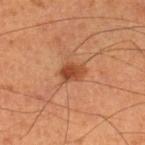Imaged during a routine full-body skin examination; the lesion was not biopsied and no histopathology is available. Approximately 3 mm at its widest. The tile uses cross-polarized illumination. A lesion tile, about 15 mm wide, cut from a 3D total-body photograph. Automated tile analysis of the lesion measured an average lesion color of about L≈48 a*≈27 b*≈37 (CIELAB) and a lesion-to-skin contrast of about 8 (normalized; higher = more distinct). The analysis additionally found a lesion-detection confidence of about 100/100. Located on the right lower leg. The patient is a male in their 60s.